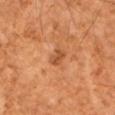follow-up=no biopsy performed (imaged during a skin exam) | diameter=~2.5 mm (longest diameter) | body site=the left upper arm | patient=male, aged approximately 55 | acquisition=total-body-photography crop, ~15 mm field of view | lighting=cross-polarized illumination.